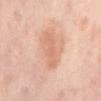- workup: imaged on a skin check; not biopsied
- location: the back
- patient: aged 53–57
- image source: total-body-photography crop, ~15 mm field of view
- tile lighting: cross-polarized illumination
- automated metrics: a footprint of about 7.5 mm², an outline eccentricity of about 0.95 (0 = round, 1 = elongated), and a symmetry-axis asymmetry near 0.35; a nevus-likeness score of about 10/100 and lesion-presence confidence of about 100/100